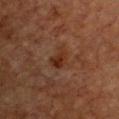| key | value |
|---|---|
| workup | imaged on a skin check; not biopsied |
| imaging modality | total-body-photography crop, ~15 mm field of view |
| subject | female, in their mid-50s |
| illumination | cross-polarized |
| size | ~3 mm (longest diameter) |
| body site | the chest |